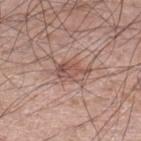lighting — white-light | patient — male, approximately 60 years of age | size — ~4 mm (longest diameter) | image-analysis metrics — an area of roughly 5.5 mm², an eccentricity of roughly 0.85, and two-axis asymmetry of about 0.4; a border-irregularity index near 4.5/10 and internal color variation of about 5 on a 0–10 scale; a lesion-detection confidence of about 100/100 | anatomic site — the left lower leg | image — total-body-photography crop, ~15 mm field of view.